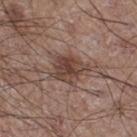workup: total-body-photography surveillance lesion; no biopsy | site: the right thigh | image source: ~15 mm tile from a whole-body skin photo | patient: male, roughly 60 years of age.